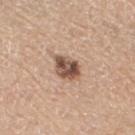size = ~3.5 mm (longest diameter); tile lighting = white-light illumination; subject = female, about 70 years old; location = the left lower leg; imaging modality = 15 mm crop, total-body photography; automated lesion analysis = a nevus-likeness score of about 75/100 and a detector confidence of about 100 out of 100 that the crop contains a lesion.